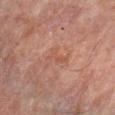Context:
A region of skin cropped from a whole-body photographic capture, roughly 15 mm wide. An algorithmic analysis of the crop reported an area of roughly 3 mm², an outline eccentricity of about 0.9 (0 = round, 1 = elongated), and a symmetry-axis asymmetry near 0.45. And it measured roughly 5 lightness units darker than nearby skin and a lesion-to-skin contrast of about 4.5 (normalized; higher = more distinct). The patient is a male aged around 60. From the left forearm.